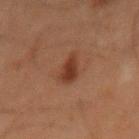follow-up=no biopsy performed (imaged during a skin exam)
site=the mid back
image=~15 mm crop, total-body skin-cancer survey
subject=male, in their mid- to late 60s